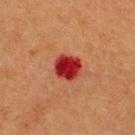Impression: This lesion was catalogued during total-body skin photography and was not selected for biopsy. Acquisition and patient details: Measured at roughly 3 mm in maximum diameter. A 15 mm crop from a total-body photograph taken for skin-cancer surveillance. On the upper back. A male subject, approximately 40 years of age. Imaged with cross-polarized lighting. Automated tile analysis of the lesion measured a lesion color around L≈31 a*≈38 b*≈29 in CIELAB, a lesion–skin lightness drop of about 15, and a lesion-to-skin contrast of about 13 (normalized; higher = more distinct). And it measured border irregularity of about 1 on a 0–10 scale and a color-variation rating of about 3.5/10. The software also gave a nevus-likeness score of about 0/100.